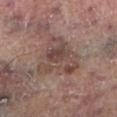The total-body-photography lesion software estimated an area of roughly 18 mm², an outline eccentricity of about 0.55 (0 = round, 1 = elongated), and two-axis asymmetry of about 0.5. The analysis additionally found border irregularity of about 6 on a 0–10 scale. It also reported a detector confidence of about 95 out of 100 that the crop contains a lesion.
A roughly 15 mm field-of-view crop from a total-body skin photograph.
From the left lower leg.
A male subject roughly 70 years of age.
Imaged with cross-polarized lighting.